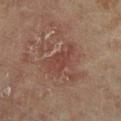workup = catalogued during a skin exam; not biopsied | illumination = cross-polarized illumination | diameter = ≈5 mm | image source = ~15 mm tile from a whole-body skin photo | location = the left lower leg | subject = female, aged around 80.